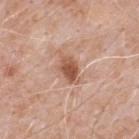| feature | finding |
|---|---|
| notes | catalogued during a skin exam; not biopsied |
| tile lighting | white-light illumination |
| acquisition | ~15 mm crop, total-body skin-cancer survey |
| site | the upper back |
| subject | male, aged around 50 |
| automated metrics | border irregularity of about 2.5 on a 0–10 scale and peripheral color asymmetry of about 1.5 |
| lesion size | about 3.5 mm |